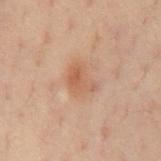  biopsy_status: not biopsied; imaged during a skin examination
  site: chest
  automated_metrics:
    area_mm2_approx: 7.0
    eccentricity: 0.65
    shape_asymmetry: 0.35
    color_variation_0_10: 3.5
    peripheral_color_asymmetry: 1.0
    nevus_likeness_0_100: 10
  image:
    source: total-body photography crop
    field_of_view_mm: 15
  patient:
    sex: male
    age_approx: 40
  lighting: cross-polarized
  lesion_size:
    long_diameter_mm_approx: 3.5